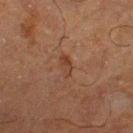follow-up=total-body-photography surveillance lesion; no biopsy | automated metrics=a nevus-likeness score of about 10/100 and a detector confidence of about 100 out of 100 that the crop contains a lesion | image source=total-body-photography crop, ~15 mm field of view | body site=the leg | subject=male, in their mid-60s | lighting=cross-polarized.